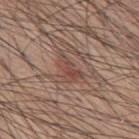Clinical impression: No biopsy was performed on this lesion — it was imaged during a full skin examination and was not determined to be concerning. Context: The lesion-visualizer software estimated a footprint of about 5.5 mm², an outline eccentricity of about 0.9 (0 = round, 1 = elongated), and a shape-asymmetry score of about 0.45 (0 = symmetric). The software also gave a mean CIELAB color near L≈46 a*≈20 b*≈24, about 7 CIELAB-L* units darker than the surrounding skin, and a normalized lesion–skin contrast near 6. The software also gave an automated nevus-likeness rating near 0 out of 100. A 15 mm crop from a total-body photograph taken for skin-cancer surveillance. A male patient, aged around 60. Located on the mid back. Approximately 4 mm at its widest.